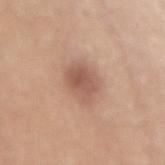notes = imaged on a skin check; not biopsied | body site = the back | size = ~4 mm (longest diameter) | imaging modality = total-body-photography crop, ~15 mm field of view | subject = male, aged approximately 55.